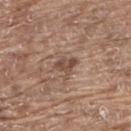notes = total-body-photography surveillance lesion; no biopsy
body site = the upper back
size = ≈3 mm
acquisition = ~15 mm tile from a whole-body skin photo
subject = male, approximately 80 years of age